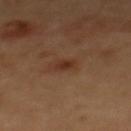workup: total-body-photography surveillance lesion; no biopsy
lesion size: ~2 mm (longest diameter)
lighting: cross-polarized
body site: the upper back
subject: female, aged 38 to 42
acquisition: total-body-photography crop, ~15 mm field of view